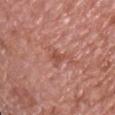workup: no biopsy performed (imaged during a skin exam) | anatomic site: the front of the torso | patient: male, approximately 75 years of age | illumination: white-light | acquisition: total-body-photography crop, ~15 mm field of view | automated metrics: a footprint of about 3.5 mm², an outline eccentricity of about 0.7 (0 = round, 1 = elongated), and a symmetry-axis asymmetry near 0.3; a lesion color around L≈51 a*≈26 b*≈29 in CIELAB, roughly 8 lightness units darker than nearby skin, and a normalized lesion–skin contrast near 6 | diameter: ~2.5 mm (longest diameter).